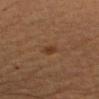The lesion was tiled from a total-body skin photograph and was not biopsied. A region of skin cropped from a whole-body photographic capture, roughly 15 mm wide. A male patient, roughly 65 years of age. Located on the left forearm.